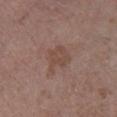Q: Is there a histopathology result?
A: imaged on a skin check; not biopsied
Q: Lesion location?
A: the right lower leg
Q: What kind of image is this?
A: ~15 mm tile from a whole-body skin photo
Q: What are the patient's age and sex?
A: female, in their mid- to late 60s